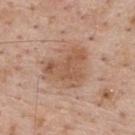This lesion was catalogued during total-body skin photography and was not selected for biopsy. Measured at roughly 6 mm in maximum diameter. Captured under white-light illumination. The patient is a male roughly 60 years of age. A close-up tile cropped from a whole-body skin photograph, about 15 mm across. On the upper back.